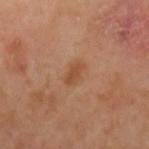workup = total-body-photography surveillance lesion; no biopsy
lesion diameter = ≈2.5 mm
site = the left upper arm
lighting = cross-polarized illumination
patient = female, aged approximately 55
imaging modality = 15 mm crop, total-body photography